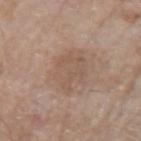Q: Is there a histopathology result?
A: total-body-photography surveillance lesion; no biopsy
Q: What is the imaging modality?
A: total-body-photography crop, ~15 mm field of view
Q: Patient demographics?
A: male, in their 80s
Q: Automated lesion metrics?
A: a shape eccentricity near 0.75; an automated nevus-likeness rating near 0 out of 100 and a lesion-detection confidence of about 100/100
Q: Illumination type?
A: white-light illumination
Q: What is the anatomic site?
A: the left upper arm